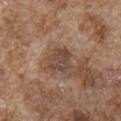Q: Is there a histopathology result?
A: total-body-photography surveillance lesion; no biopsy
Q: What did automated image analysis measure?
A: an area of roughly 7.5 mm², an eccentricity of roughly 0.45, and a shape-asymmetry score of about 0.2 (0 = symmetric); a lesion color around L≈44 a*≈17 b*≈25 in CIELAB, a lesion–skin lightness drop of about 9, and a lesion-to-skin contrast of about 7 (normalized; higher = more distinct); a border-irregularity rating of about 2.5/10 and peripheral color asymmetry of about 1; a classifier nevus-likeness of about 5/100 and lesion-presence confidence of about 100/100
Q: Patient demographics?
A: male, aged approximately 75
Q: What is the lesion's diameter?
A: ~3 mm (longest diameter)
Q: Illumination type?
A: white-light
Q: What is the anatomic site?
A: the right upper arm
Q: What kind of image is this?
A: total-body-photography crop, ~15 mm field of view